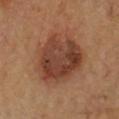<lesion>
<biopsy_status>not biopsied; imaged during a skin examination</biopsy_status>
<image>
  <source>total-body photography crop</source>
  <field_of_view_mm>15</field_of_view_mm>
</image>
<lesion_size>
  <long_diameter_mm_approx>6.5</long_diameter_mm_approx>
</lesion_size>
<patient>
  <sex>male</sex>
  <age_approx>55</age_approx>
</patient>
<site>chest</site>
<lighting>cross-polarized</lighting>
</lesion>